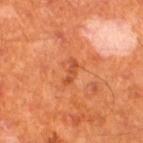Clinical impression:
Imaged during a routine full-body skin examination; the lesion was not biopsied and no histopathology is available.
Context:
A close-up tile cropped from a whole-body skin photograph, about 15 mm across. A male subject, aged approximately 60. The lesion is on the left thigh. Longest diameter approximately 3 mm.